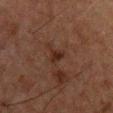Impression: Recorded during total-body skin imaging; not selected for excision or biopsy. Background: The patient is a male approximately 50 years of age. The lesion is on the chest. A 15 mm crop from a total-body photograph taken for skin-cancer surveillance.